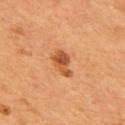Q: Is there a histopathology result?
A: catalogued during a skin exam; not biopsied
Q: Who is the patient?
A: male, aged approximately 55
Q: What is the anatomic site?
A: the back
Q: What is the imaging modality?
A: ~15 mm tile from a whole-body skin photo
Q: Lesion size?
A: about 4 mm
Q: What did automated image analysis measure?
A: an area of roughly 6 mm² and a shape-asymmetry score of about 0.3 (0 = symmetric); a mean CIELAB color near L≈50 a*≈26 b*≈39, roughly 11 lightness units darker than nearby skin, and a lesion-to-skin contrast of about 8 (normalized; higher = more distinct); a border-irregularity rating of about 3.5/10, internal color variation of about 4.5 on a 0–10 scale, and a peripheral color-asymmetry measure near 1.5; a lesion-detection confidence of about 100/100